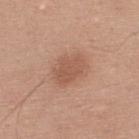Captured during whole-body skin photography for melanoma surveillance; the lesion was not biopsied.
A male subject, about 30 years old.
Captured under white-light illumination.
The lesion-visualizer software estimated a footprint of about 8.5 mm², a shape eccentricity near 0.8, and a symmetry-axis asymmetry near 0.3. And it measured a border-irregularity rating of about 3/10, a color-variation rating of about 2/10, and a peripheral color-asymmetry measure near 0.5. The analysis additionally found a nevus-likeness score of about 60/100.
A lesion tile, about 15 mm wide, cut from a 3D total-body photograph.
Located on the upper back.